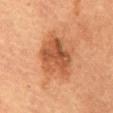Impression:
Imaged during a routine full-body skin examination; the lesion was not biopsied and no histopathology is available.
Background:
Located on the abdomen. The lesion-visualizer software estimated a within-lesion color-variation index near 5.5/10. And it measured a nevus-likeness score of about 50/100 and a lesion-detection confidence of about 100/100. A 15 mm crop from a total-body photograph taken for skin-cancer surveillance. Imaged with cross-polarized lighting. Longest diameter approximately 5 mm. A female subject, aged 68 to 72.